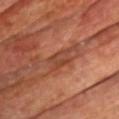Imaged during a routine full-body skin examination; the lesion was not biopsied and no histopathology is available.
On the chest.
The subject is a male aged approximately 60.
The tile uses cross-polarized illumination.
A close-up tile cropped from a whole-body skin photograph, about 15 mm across.